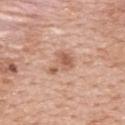Q: Was this lesion biopsied?
A: imaged on a skin check; not biopsied
Q: What is the imaging modality?
A: ~15 mm tile from a whole-body skin photo
Q: How was the tile lit?
A: white-light illumination
Q: What is the anatomic site?
A: the upper back
Q: What are the patient's age and sex?
A: female, aged around 60
Q: Automated lesion metrics?
A: an average lesion color of about L≈59 a*≈23 b*≈31 (CIELAB), a lesion–skin lightness drop of about 11, and a lesion-to-skin contrast of about 7 (normalized; higher = more distinct); border irregularity of about 6.5 on a 0–10 scale, a color-variation rating of about 3/10, and radial color variation of about 1; a classifier nevus-likeness of about 30/100